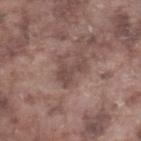| feature | finding |
|---|---|
| notes | total-body-photography surveillance lesion; no biopsy |